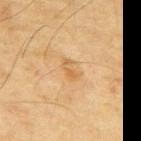Clinical impression: The lesion was photographed on a routine skin check and not biopsied; there is no pathology result. Clinical summary: Cropped from a whole-body photographic skin survey; the tile spans about 15 mm. The tile uses cross-polarized illumination. A male subject aged around 70. The lesion is located on the upper back.